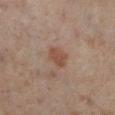Assessment:
Part of a total-body skin-imaging series; this lesion was reviewed on a skin check and was not flagged for biopsy.
Context:
This is a cross-polarized tile. The lesion is located on the right lower leg. About 2.5 mm across. The patient is a female aged 48–52. A region of skin cropped from a whole-body photographic capture, roughly 15 mm wide.